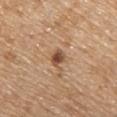notes: catalogued during a skin exam; not biopsied
patient: male, roughly 70 years of age
image source: ~15 mm crop, total-body skin-cancer survey
size: about 2.5 mm
illumination: white-light illumination
location: the chest